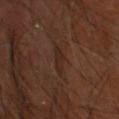This lesion was catalogued during total-body skin photography and was not selected for biopsy.
On the head or neck.
This image is a 15 mm lesion crop taken from a total-body photograph.
The subject is a male about 60 years old.